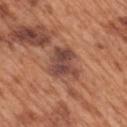Captured during whole-body skin photography for melanoma surveillance; the lesion was not biopsied. Cropped from a whole-body photographic skin survey; the tile spans about 15 mm. The total-body-photography lesion software estimated a footprint of about 12 mm² and a symmetry-axis asymmetry near 0.3. The software also gave about 11 CIELAB-L* units darker than the surrounding skin and a normalized lesion–skin contrast near 9.5. The software also gave a border-irregularity rating of about 3.5/10, internal color variation of about 4.5 on a 0–10 scale, and a peripheral color-asymmetry measure near 1.5. It also reported a nevus-likeness score of about 0/100 and a detector confidence of about 100 out of 100 that the crop contains a lesion. Imaged with white-light lighting. The lesion is on the mid back. Measured at roughly 4 mm in maximum diameter. The subject is a male aged approximately 65.